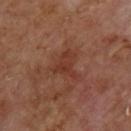Case summary:
– biopsy status — no biopsy performed (imaged during a skin exam)
– illumination — cross-polarized illumination
– subject — male, roughly 70 years of age
– lesion size — about 3.5 mm
– acquisition — 15 mm crop, total-body photography
– site — the upper back